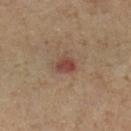Q: Was this lesion biopsied?
A: no biopsy performed (imaged during a skin exam)
Q: What is the imaging modality?
A: ~15 mm tile from a whole-body skin photo
Q: What is the lesion's diameter?
A: ≈3 mm
Q: What is the anatomic site?
A: the left lower leg
Q: Who is the patient?
A: male, in their mid- to late 50s
Q: What lighting was used for the tile?
A: cross-polarized